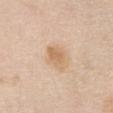Captured during whole-body skin photography for melanoma surveillance; the lesion was not biopsied.
A female patient aged approximately 70.
A 15 mm close-up extracted from a 3D total-body photography capture.
The total-body-photography lesion software estimated a border-irregularity index near 2/10, a color-variation rating of about 3/10, and radial color variation of about 1.
From the chest.